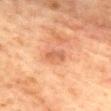This lesion was catalogued during total-body skin photography and was not selected for biopsy. Automated tile analysis of the lesion measured a lesion color around L≈49 a*≈22 b*≈32 in CIELAB and a normalized lesion–skin contrast near 6. The analysis additionally found border irregularity of about 3.5 on a 0–10 scale, internal color variation of about 1 on a 0–10 scale, and peripheral color asymmetry of about 0. And it measured a nevus-likeness score of about 0/100 and a detector confidence of about 100 out of 100 that the crop contains a lesion. The lesion is on the front of the torso. The tile uses cross-polarized illumination. A 15 mm close-up extracted from a 3D total-body photography capture. The subject is a female aged 58 to 62. Measured at roughly 2.5 mm in maximum diameter.